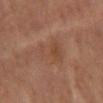Notes:
– notes: catalogued during a skin exam; not biopsied
– subject: male, roughly 60 years of age
– location: the mid back
– lesion size: ~4.5 mm (longest diameter)
– acquisition: ~15 mm tile from a whole-body skin photo